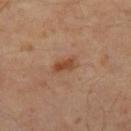tile lighting = cross-polarized | patient = male, aged approximately 55 | anatomic site = the right thigh | imaging modality = 15 mm crop, total-body photography | lesion size = about 3 mm.